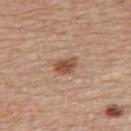Assessment: The lesion was tiled from a total-body skin photograph and was not biopsied. Clinical summary: Imaged with white-light lighting. Automated image analysis of the tile measured a footprint of about 4.5 mm², an outline eccentricity of about 0.75 (0 = round, 1 = elongated), and two-axis asymmetry of about 0.25. It also reported an average lesion color of about L≈51 a*≈21 b*≈31 (CIELAB) and a lesion–skin lightness drop of about 12. It also reported a border-irregularity index near 2/10, a within-lesion color-variation index near 3/10, and peripheral color asymmetry of about 1. The analysis additionally found a nevus-likeness score of about 90/100 and a lesion-detection confidence of about 100/100. The lesion is located on the upper back. A female subject approximately 60 years of age. Approximately 3 mm at its widest. A 15 mm crop from a total-body photograph taken for skin-cancer surveillance.